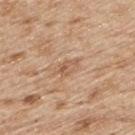Q: Is there a histopathology result?
A: catalogued during a skin exam; not biopsied